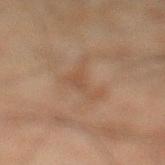Q: Is there a histopathology result?
A: no biopsy performed (imaged during a skin exam)
Q: Who is the patient?
A: male, aged approximately 45
Q: Lesion size?
A: about 5.5 mm
Q: How was this image acquired?
A: ~15 mm tile from a whole-body skin photo
Q: Where on the body is the lesion?
A: the left lower leg